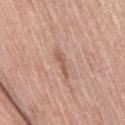{
  "patient": {
    "sex": "female",
    "age_approx": 60
  },
  "image": {
    "source": "total-body photography crop",
    "field_of_view_mm": 15
  },
  "site": "leg",
  "automated_metrics": {
    "area_mm2_approx": 3.0,
    "eccentricity": 0.95,
    "nevus_likeness_0_100": 0,
    "lesion_detection_confidence_0_100": 95
  },
  "lesion_size": {
    "long_diameter_mm_approx": 3.5
  },
  "lighting": "white-light"
}